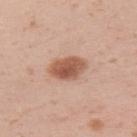A 15 mm close-up extracted from a 3D total-body photography capture. Located on the left upper arm. The patient is a female aged 18 to 22. Longest diameter approximately 4.5 mm. This is a white-light tile.